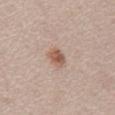Recorded during total-body skin imaging; not selected for excision or biopsy.
The lesion-visualizer software estimated a footprint of about 4 mm². And it measured a lesion color around L≈56 a*≈20 b*≈28 in CIELAB, about 12 CIELAB-L* units darker than the surrounding skin, and a normalized border contrast of about 8.5. And it measured border irregularity of about 2 on a 0–10 scale, a within-lesion color-variation index near 4/10, and a peripheral color-asymmetry measure near 1.5. The software also gave a classifier nevus-likeness of about 95/100 and a lesion-detection confidence of about 100/100.
A lesion tile, about 15 mm wide, cut from a 3D total-body photograph.
A male patient in their mid- to late 50s.
The recorded lesion diameter is about 2.5 mm.
The lesion is on the mid back.